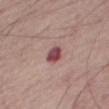Q: Was this lesion biopsied?
A: catalogued during a skin exam; not biopsied
Q: How was this image acquired?
A: total-body-photography crop, ~15 mm field of view
Q: How was the tile lit?
A: white-light
Q: Automated lesion metrics?
A: a footprint of about 4.5 mm², an eccentricity of roughly 0.65, and a shape-asymmetry score of about 0.3 (0 = symmetric); a border-irregularity index near 2/10, a within-lesion color-variation index near 4.5/10, and peripheral color asymmetry of about 1.5; a nevus-likeness score of about 0/100 and lesion-presence confidence of about 100/100
Q: Where on the body is the lesion?
A: the right thigh
Q: What is the lesion's diameter?
A: ~2.5 mm (longest diameter)
Q: What are the patient's age and sex?
A: male, about 75 years old